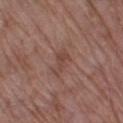No biopsy was performed on this lesion — it was imaged during a full skin examination and was not determined to be concerning. On the right thigh. Cropped from a total-body skin-imaging series; the visible field is about 15 mm. A female patient aged 68–72.